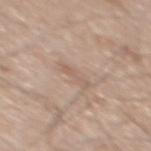Clinical summary:
A male patient about 65 years old. Located on the mid back. This image is a 15 mm lesion crop taken from a total-body photograph.